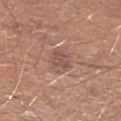Assessment:
Captured during whole-body skin photography for melanoma surveillance; the lesion was not biopsied.
Clinical summary:
Imaged with white-light lighting. A male subject aged 78 to 82. The lesion is located on the right lower leg. The recorded lesion diameter is about 2.5 mm. This image is a 15 mm lesion crop taken from a total-body photograph.